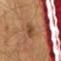{"biopsy_status": "not biopsied; imaged during a skin examination", "site": "abdomen", "lighting": "cross-polarized", "automated_metrics": {"cielab_L": 40, "cielab_a": 20, "cielab_b": 30, "vs_skin_darker_L": 9.0, "vs_skin_contrast_norm": 7.5, "border_irregularity_0_10": 4.5, "color_variation_0_10": 2.0, "peripheral_color_asymmetry": 1.0, "nevus_likeness_0_100": 85, "lesion_detection_confidence_0_100": 100}, "lesion_size": {"long_diameter_mm_approx": 4.0}, "patient": {"sex": "male", "age_approx": 60}, "image": {"source": "total-body photography crop", "field_of_view_mm": 15}}